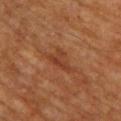Located on the upper back. The recorded lesion diameter is about 4 mm. Imaged with cross-polarized lighting. A male subject, aged 58–62. Cropped from a whole-body photographic skin survey; the tile spans about 15 mm.